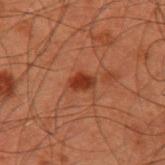– workup — total-body-photography surveillance lesion; no biopsy
– site — the left upper arm
– subject — male, in their 60s
– imaging modality — 15 mm crop, total-body photography